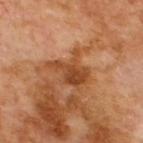• TBP lesion metrics: an automated nevus-likeness rating near 0 out of 100
• tile lighting: cross-polarized illumination
• image: 15 mm crop, total-body photography
• anatomic site: the upper back
• diameter: ≈4.5 mm
• patient: male, aged around 70